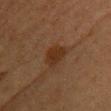biopsy status: no biopsy performed (imaged during a skin exam) | acquisition: ~15 mm crop, total-body skin-cancer survey | location: the front of the torso | diameter: ~3.5 mm (longest diameter) | subject: female, about 55 years old | automated lesion analysis: a footprint of about 6.5 mm², an outline eccentricity of about 0.75 (0 = round, 1 = elongated), and two-axis asymmetry of about 0.25; a classifier nevus-likeness of about 40/100 and lesion-presence confidence of about 100/100 | illumination: cross-polarized.